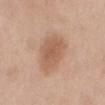Recorded during total-body skin imaging; not selected for excision or biopsy.
An algorithmic analysis of the crop reported an outline eccentricity of about 0.8 (0 = round, 1 = elongated) and two-axis asymmetry of about 0.2. The analysis additionally found a border-irregularity rating of about 2/10, internal color variation of about 2.5 on a 0–10 scale, and radial color variation of about 0.5. The analysis additionally found a detector confidence of about 100 out of 100 that the crop contains a lesion.
A female patient, approximately 60 years of age.
A lesion tile, about 15 mm wide, cut from a 3D total-body photograph.
Longest diameter approximately 5.5 mm.
The lesion is located on the mid back.